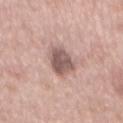Assessment:
This lesion was catalogued during total-body skin photography and was not selected for biopsy.
Clinical summary:
The lesion is on the mid back. The lesion's longest dimension is about 4.5 mm. Captured under white-light illumination. A male patient aged 63–67. Cropped from a total-body skin-imaging series; the visible field is about 15 mm.